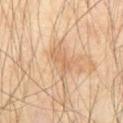Q: Was a biopsy performed?
A: total-body-photography surveillance lesion; no biopsy
Q: What are the patient's age and sex?
A: male, aged 68 to 72
Q: Where on the body is the lesion?
A: the abdomen
Q: What is the imaging modality?
A: ~15 mm tile from a whole-body skin photo
Q: What lighting was used for the tile?
A: cross-polarized
Q: Lesion size?
A: about 3 mm